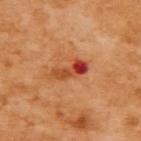The lesion was photographed on a routine skin check and not biopsied; there is no pathology result. This image is a 15 mm lesion crop taken from a total-body photograph. The subject is a female aged approximately 55. On the back. The lesion's longest dimension is about 4.5 mm.